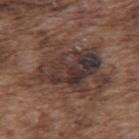workup = catalogued during a skin exam; not biopsied | diameter = ~9.5 mm (longest diameter) | site = the upper back | tile lighting = white-light | imaging modality = total-body-photography crop, ~15 mm field of view | patient = male, aged 73–77.